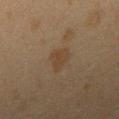Imaged during a routine full-body skin examination; the lesion was not biopsied and no histopathology is available. From the right upper arm. The patient is a female aged around 40. The tile uses cross-polarized illumination. A region of skin cropped from a whole-body photographic capture, roughly 15 mm wide.